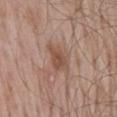Assessment:
Imaged during a routine full-body skin examination; the lesion was not biopsied and no histopathology is available.
Clinical summary:
The lesion's longest dimension is about 4 mm. Automated tile analysis of the lesion measured a lesion area of about 6 mm², an eccentricity of roughly 0.8, and a shape-asymmetry score of about 0.4 (0 = symmetric). The analysis additionally found an average lesion color of about L≈50 a*≈19 b*≈28 (CIELAB). It also reported radial color variation of about 1. The software also gave a classifier nevus-likeness of about 5/100 and lesion-presence confidence of about 100/100. A male patient, in their mid-60s. This is a white-light tile. A 15 mm crop from a total-body photograph taken for skin-cancer surveillance. The lesion is on the mid back.